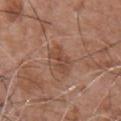biopsy_status: not biopsied; imaged during a skin examination
site: chest
patient:
  sex: male
  age_approx: 75
image:
  source: total-body photography crop
  field_of_view_mm: 15